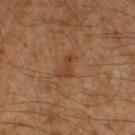Q: Is there a histopathology result?
A: imaged on a skin check; not biopsied
Q: Who is the patient?
A: male, aged 58–62
Q: What lighting was used for the tile?
A: cross-polarized illumination
Q: Lesion location?
A: the left lower leg
Q: What kind of image is this?
A: ~15 mm tile from a whole-body skin photo
Q: Lesion size?
A: ≈3 mm